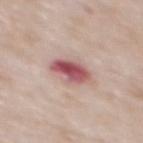Captured during whole-body skin photography for melanoma surveillance; the lesion was not biopsied. A 15 mm close-up tile from a total-body photography series done for melanoma screening. The patient is a male about 65 years old. The lesion's longest dimension is about 3.5 mm. On the mid back. Captured under white-light illumination.